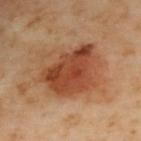Q: Was a biopsy performed?
A: catalogued during a skin exam; not biopsied
Q: How was the tile lit?
A: cross-polarized
Q: Where on the body is the lesion?
A: the upper back
Q: Lesion size?
A: about 7.5 mm
Q: What are the patient's age and sex?
A: female, aged 58–62
Q: What kind of image is this?
A: ~15 mm tile from a whole-body skin photo
Q: What did automated image analysis measure?
A: a border-irregularity index near 3/10, a color-variation rating of about 6.5/10, and peripheral color asymmetry of about 2; an automated nevus-likeness rating near 90 out of 100 and a detector confidence of about 100 out of 100 that the crop contains a lesion